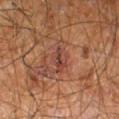Clinical impression: Imaged during a routine full-body skin examination; the lesion was not biopsied and no histopathology is available. Image and clinical context: The lesion's longest dimension is about 3.5 mm. Imaged with cross-polarized lighting. A roughly 15 mm field-of-view crop from a total-body skin photograph. From the right leg. A male subject aged approximately 60.